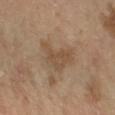Clinical impression: Recorded during total-body skin imaging; not selected for excision or biopsy. Acquisition and patient details: The subject is a female aged approximately 60. Cropped from a whole-body photographic skin survey; the tile spans about 15 mm. The lesion is on the right forearm. The lesion's longest dimension is about 5 mm. The tile uses cross-polarized illumination.